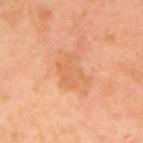The lesion was tiled from a total-body skin photograph and was not biopsied. About 4 mm across. Cropped from a total-body skin-imaging series; the visible field is about 15 mm. The tile uses cross-polarized illumination. The patient is a female in their mid- to late 50s. Located on the right upper arm. The total-body-photography lesion software estimated an average lesion color of about L≈67 a*≈26 b*≈41 (CIELAB) and a lesion-to-skin contrast of about 4.5 (normalized; higher = more distinct). It also reported a lesion-detection confidence of about 100/100.